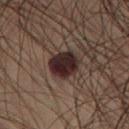Clinical impression:
The lesion was tiled from a total-body skin photograph and was not biopsied.
Acquisition and patient details:
A male patient aged around 50. Captured under cross-polarized illumination. A roughly 15 mm field-of-view crop from a total-body skin photograph. Approximately 3.5 mm at its widest. The lesion is located on the left thigh.